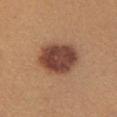* notes: no biopsy performed (imaged during a skin exam)
* subject: female, in their mid- to late 20s
* imaging modality: ~15 mm crop, total-body skin-cancer survey
* body site: the chest
* lesion size: about 5.5 mm
* image-analysis metrics: a color-variation rating of about 4.5/10 and a peripheral color-asymmetry measure near 1; an automated nevus-likeness rating near 90 out of 100 and a lesion-detection confidence of about 100/100
* tile lighting: white-light